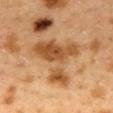Notes:
- follow-up — no biopsy performed (imaged during a skin exam)
- site — the mid back
- image source — ~15 mm crop, total-body skin-cancer survey
- subject — female, in their 40s
- illumination — cross-polarized
- automated lesion analysis — an outline eccentricity of about 0.65 (0 = round, 1 = elongated) and a symmetry-axis asymmetry near 0.7; an average lesion color of about L≈43 a*≈19 b*≈34 (CIELAB), a lesion–skin lightness drop of about 11, and a lesion-to-skin contrast of about 9 (normalized; higher = more distinct); a border-irregularity index near 9/10, internal color variation of about 4.5 on a 0–10 scale, and a peripheral color-asymmetry measure near 1.5
- lesion size — ~7 mm (longest diameter)